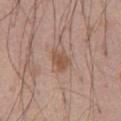Part of a total-body skin-imaging series; this lesion was reviewed on a skin check and was not flagged for biopsy.
This is a white-light tile.
From the abdomen.
This image is a 15 mm lesion crop taken from a total-body photograph.
A male patient, about 60 years old.